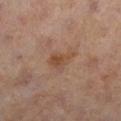notes: no biopsy performed (imaged during a skin exam)
lighting: cross-polarized illumination
subject: female, aged 48–52
TBP lesion metrics: a lesion area of about 4 mm²; an average lesion color of about L≈45 a*≈19 b*≈30 (CIELAB), roughly 7 lightness units darker than nearby skin, and a normalized border contrast of about 6.5; border irregularity of about 5 on a 0–10 scale, a within-lesion color-variation index near 2/10, and a peripheral color-asymmetry measure near 0.5
lesion size: ~3 mm (longest diameter)
image: total-body-photography crop, ~15 mm field of view
body site: the right lower leg